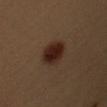Assessment: Part of a total-body skin-imaging series; this lesion was reviewed on a skin check and was not flagged for biopsy. Image and clinical context: This is a cross-polarized tile. The lesion is on the left upper arm. A close-up tile cropped from a whole-body skin photograph, about 15 mm across. The patient is a female aged 43–47. Automated image analysis of the tile measured a lesion color around L≈23 a*≈17 b*≈22 in CIELAB, a lesion–skin lightness drop of about 11, and a normalized lesion–skin contrast near 12.